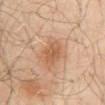* workup — catalogued during a skin exam; not biopsied